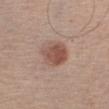biopsy status — catalogued during a skin exam; not biopsied | lesion size — ~4 mm (longest diameter) | site — the right upper arm | acquisition — ~15 mm tile from a whole-body skin photo | patient — male, in their mid- to late 50s.